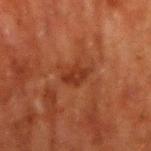Imaged during a routine full-body skin examination; the lesion was not biopsied and no histopathology is available.
The recorded lesion diameter is about 3 mm.
Cropped from a total-body skin-imaging series; the visible field is about 15 mm.
The tile uses cross-polarized illumination.
The lesion is on the arm.
A male subject, aged 58–62.